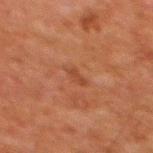notes = no biopsy performed (imaged during a skin exam); site = the mid back; diameter = about 2.5 mm; subject = male, approximately 80 years of age; imaging modality = 15 mm crop, total-body photography.